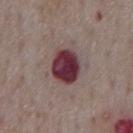* notes: total-body-photography surveillance lesion; no biopsy
* lesion size: ≈4 mm
* image: ~15 mm crop, total-body skin-cancer survey
* illumination: white-light illumination
* location: the chest
* subject: male, approximately 75 years of age